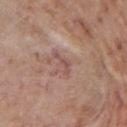workup: catalogued during a skin exam; not biopsied
site: the chest
image source: ~15 mm crop, total-body skin-cancer survey
diameter: ≈3 mm
automated metrics: a lesion area of about 2.5 mm², an outline eccentricity of about 0.95 (0 = round, 1 = elongated), and a shape-asymmetry score of about 0.6 (0 = symmetric); an automated nevus-likeness rating near 0 out of 100 and a detector confidence of about 85 out of 100 that the crop contains a lesion
subject: male, roughly 55 years of age